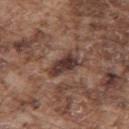follow-up — catalogued during a skin exam; not biopsied
body site — the upper back
illumination — white-light
lesion size — ≈4 mm
subject — male, aged 73–77
imaging modality — 15 mm crop, total-body photography